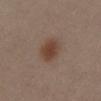Assessment: No biopsy was performed on this lesion — it was imaged during a full skin examination and was not determined to be concerning. Context: A female subject aged approximately 30. The total-body-photography lesion software estimated an area of roughly 8.5 mm² and two-axis asymmetry of about 0.2. This is a white-light tile. From the abdomen. A 15 mm close-up tile from a total-body photography series done for melanoma screening.